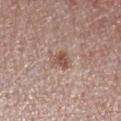Captured during whole-body skin photography for melanoma surveillance; the lesion was not biopsied.
Located on the arm.
The patient is a female aged 48–52.
A region of skin cropped from a whole-body photographic capture, roughly 15 mm wide.